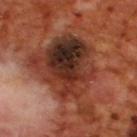The lesion was tiled from a total-body skin photograph and was not biopsied. The lesion is located on the upper back. Captured under cross-polarized illumination. A 15 mm close-up extracted from a 3D total-body photography capture. A male subject, aged approximately 70.